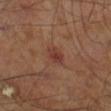Clinical impression: The lesion was tiled from a total-body skin photograph and was not biopsied. Acquisition and patient details: The subject is a male approximately 60 years of age. Captured under cross-polarized illumination. The lesion is located on the right leg. This image is a 15 mm lesion crop taken from a total-body photograph. An algorithmic analysis of the crop reported a classifier nevus-likeness of about 5/100 and lesion-presence confidence of about 100/100. The lesion's longest dimension is about 2.5 mm.